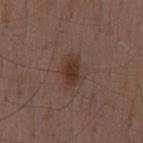Notes:
– biopsy status: total-body-photography surveillance lesion; no biopsy
– illumination: white-light illumination
– body site: the lower back
– automated lesion analysis: a border-irregularity index near 2/10, a color-variation rating of about 2.5/10, and radial color variation of about 1; a detector confidence of about 100 out of 100 that the crop contains a lesion
– image: 15 mm crop, total-body photography
– patient: male, about 50 years old
– lesion diameter: ≈3.5 mm